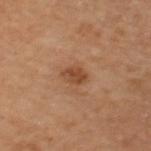Impression: The lesion was tiled from a total-body skin photograph and was not biopsied. Acquisition and patient details: Located on the right thigh. A male subject, about 65 years old. A region of skin cropped from a whole-body photographic capture, roughly 15 mm wide.